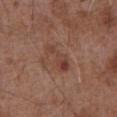Imaged during a routine full-body skin examination; the lesion was not biopsied and no histopathology is available.
Automated image analysis of the tile measured an eccentricity of roughly 0.9 and two-axis asymmetry of about 0.55. The software also gave an average lesion color of about L≈41 a*≈22 b*≈26 (CIELAB) and a normalized border contrast of about 6. And it measured a border-irregularity index near 6/10, a within-lesion color-variation index near 5.5/10, and peripheral color asymmetry of about 1.
The recorded lesion diameter is about 4 mm.
A 15 mm close-up tile from a total-body photography series done for melanoma screening.
A male patient aged 73–77.
Located on the abdomen.